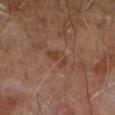workup = no biopsy performed (imaged during a skin exam)
image = total-body-photography crop, ~15 mm field of view
lighting = cross-polarized
lesion size = ~3 mm (longest diameter)
subject = male, aged 63 to 67